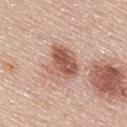biopsy_status: not biopsied; imaged during a skin examination